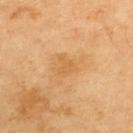notes: imaged on a skin check; not biopsied
subject: male, approximately 70 years of age
acquisition: total-body-photography crop, ~15 mm field of view
site: the upper back
automated metrics: an area of roughly 4 mm² and a shape-asymmetry score of about 0.55 (0 = symmetric); a border-irregularity index near 5/10, a color-variation rating of about 0.5/10, and a peripheral color-asymmetry measure near 0; a nevus-likeness score of about 0/100 and lesion-presence confidence of about 100/100
size: about 2.5 mm
lighting: cross-polarized